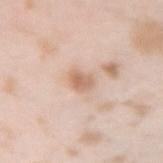Notes:
– notes — imaged on a skin check; not biopsied
– acquisition — ~15 mm tile from a whole-body skin photo
– body site — the right forearm
– patient — female, aged 23–27
– lighting — white-light illumination
– automated metrics — an area of roughly 4.5 mm², an eccentricity of roughly 0.6, and a shape-asymmetry score of about 0.2 (0 = symmetric); a mean CIELAB color near L≈66 a*≈19 b*≈30 and a lesion–skin lightness drop of about 10